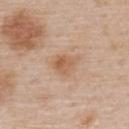notes: no biopsy performed (imaged during a skin exam); image source: ~15 mm crop, total-body skin-cancer survey; lesion diameter: ~3.5 mm (longest diameter); body site: the upper back; tile lighting: white-light illumination; patient: male, aged around 60.